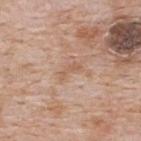Imaged during a routine full-body skin examination; the lesion was not biopsied and no histopathology is available.
The tile uses white-light illumination.
A male subject, aged 63–67.
The lesion's longest dimension is about 3 mm.
A close-up tile cropped from a whole-body skin photograph, about 15 mm across.
The lesion is on the upper back.